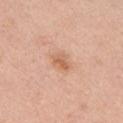<lesion>
  <biopsy_status>not biopsied; imaged during a skin examination</biopsy_status>
  <lighting>white-light</lighting>
  <automated_metrics>
    <area_mm2_approx>3.5</area_mm2_approx>
    <eccentricity>0.75</eccentricity>
    <shape_asymmetry>0.2</shape_asymmetry>
    <cielab_L>62</cielab_L>
    <cielab_a>23</cielab_a>
    <cielab_b>34</cielab_b>
    <border_irregularity_0_10>2.0</border_irregularity_0_10>
    <color_variation_0_10>3.0</color_variation_0_10>
  </automated_metrics>
  <patient>
    <sex>female</sex>
    <age_approx>45</age_approx>
  </patient>
  <image>
    <source>total-body photography crop</source>
    <field_of_view_mm>15</field_of_view_mm>
  </image>
  <site>chest</site>
</lesion>